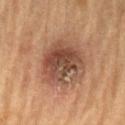Clinical impression: This lesion was catalogued during total-body skin photography and was not selected for biopsy. Image and clinical context: A male subject aged approximately 85 or older. The lesion's longest dimension is about 6.5 mm. Located on the lower back. A 15 mm close-up tile from a total-body photography series done for melanoma screening.